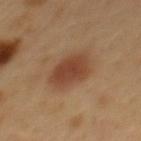tile lighting: cross-polarized | patient: male, aged 48 to 52 | automated lesion analysis: border irregularity of about 1.5 on a 0–10 scale, internal color variation of about 3 on a 0–10 scale, and a peripheral color-asymmetry measure near 1; a classifier nevus-likeness of about 100/100 | site: the back | diameter: ~4.5 mm (longest diameter) | image: ~15 mm crop, total-body skin-cancer survey.